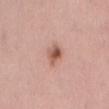biopsy_status: not biopsied; imaged during a skin examination
patient:
  sex: female
  age_approx: 65
site: mid back
lighting: white-light
automated_metrics:
  area_mm2_approx: 4.5
  eccentricity: 0.75
  shape_asymmetry: 0.25
  cielab_L: 56
  cielab_a: 23
  cielab_b: 28
  vs_skin_darker_L: 13.0
  vs_skin_contrast_norm: 8.5
  nevus_likeness_0_100: 85
  lesion_detection_confidence_0_100: 100
image:
  source: total-body photography crop
  field_of_view_mm: 15
lesion_size:
  long_diameter_mm_approx: 3.0